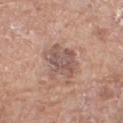notes = imaged on a skin check; not biopsied | lesion diameter = about 5 mm | imaging modality = total-body-photography crop, ~15 mm field of view | body site = the left thigh | tile lighting = white-light illumination | subject = female, about 75 years old.